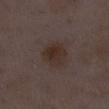  biopsy_status: not biopsied; imaged during a skin examination
  automated_metrics:
    area_mm2_approx: 9.0
    eccentricity: 0.4
    shape_asymmetry: 0.15
    cielab_L: 29
    cielab_a: 13
    cielab_b: 19
    vs_skin_darker_L: 6.0
    vs_skin_contrast_norm: 8.0
    border_irregularity_0_10: 1.5
    color_variation_0_10: 3.5
    peripheral_color_asymmetry: 1.0
  image:
    source: total-body photography crop
    field_of_view_mm: 15
  lesion_size:
    long_diameter_mm_approx: 3.5
  site: right lower leg
  patient:
    sex: female
    age_approx: 30